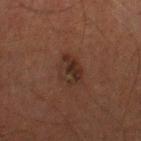This lesion was catalogued during total-body skin photography and was not selected for biopsy.
The total-body-photography lesion software estimated an average lesion color of about L≈23 a*≈14 b*≈20 (CIELAB), about 6 CIELAB-L* units darker than the surrounding skin, and a normalized border contrast of about 7.
On the left lower leg.
A male subject aged 63 to 67.
The recorded lesion diameter is about 4 mm.
A roughly 15 mm field-of-view crop from a total-body skin photograph.